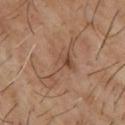{
  "biopsy_status": "not biopsied; imaged during a skin examination",
  "image": {
    "source": "total-body photography crop",
    "field_of_view_mm": 15
  },
  "automated_metrics": {
    "eccentricity": 0.95,
    "shape_asymmetry": 0.45,
    "cielab_L": 46,
    "cielab_a": 17,
    "cielab_b": 27,
    "vs_skin_darker_L": 6.0,
    "vs_skin_contrast_norm": 5.0,
    "border_irregularity_0_10": 8.5,
    "color_variation_0_10": 4.5,
    "peripheral_color_asymmetry": 1.5,
    "lesion_detection_confidence_0_100": 85
  },
  "patient": {
    "sex": "male",
    "age_approx": 65
  },
  "site": "upper back",
  "lighting": "cross-polarized"
}